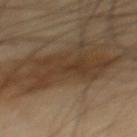Case summary:
* follow-up: catalogued during a skin exam; not biopsied
* image source: total-body-photography crop, ~15 mm field of view
* patient: male, aged approximately 65
* size: ~8.5 mm (longest diameter)
* illumination: cross-polarized illumination
* anatomic site: the mid back